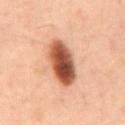Findings:
– workup · catalogued during a skin exam; not biopsied
– imaging modality · total-body-photography crop, ~15 mm field of view
– subject · male, roughly 60 years of age
– anatomic site · the abdomen
– TBP lesion metrics · a border-irregularity index near 1/10, a within-lesion color-variation index near 8/10, and a peripheral color-asymmetry measure near 2.5
– diameter · about 5.5 mm